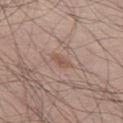No biopsy was performed on this lesion — it was imaged during a full skin examination and was not determined to be concerning. The recorded lesion diameter is about 2.5 mm. A male subject aged 48–52. Captured under white-light illumination. Located on the left thigh. Cropped from a whole-body photographic skin survey; the tile spans about 15 mm.